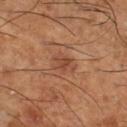Captured during whole-body skin photography for melanoma surveillance; the lesion was not biopsied.